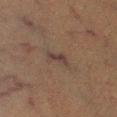Part of a total-body skin-imaging series; this lesion was reviewed on a skin check and was not flagged for biopsy. Located on the right lower leg. A close-up tile cropped from a whole-body skin photograph, about 15 mm across. A male subject, roughly 50 years of age. Longest diameter approximately 3 mm. Automated tile analysis of the lesion measured a footprint of about 4 mm², an outline eccentricity of about 0.9 (0 = round, 1 = elongated), and a symmetry-axis asymmetry near 0.3. The software also gave a mean CIELAB color near L≈30 a*≈13 b*≈17 and a normalized lesion–skin contrast near 7. Captured under cross-polarized illumination.